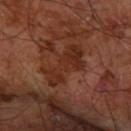Clinical impression: Part of a total-body skin-imaging series; this lesion was reviewed on a skin check and was not flagged for biopsy. Acquisition and patient details: A male patient aged approximately 60. The lesion is located on the arm. Imaged with cross-polarized lighting. A lesion tile, about 15 mm wide, cut from a 3D total-body photograph. Measured at roughly 6 mm in maximum diameter.